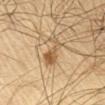Q: Is there a histopathology result?
A: catalogued during a skin exam; not biopsied
Q: Automated lesion metrics?
A: a lesion area of about 6.5 mm², an outline eccentricity of about 0.9 (0 = round, 1 = elongated), and a symmetry-axis asymmetry near 0.45; a lesion color around L≈56 a*≈16 b*≈36 in CIELAB, about 11 CIELAB-L* units darker than the surrounding skin, and a normalized border contrast of about 7.5
Q: What is the imaging modality?
A: 15 mm crop, total-body photography
Q: How large is the lesion?
A: about 4.5 mm
Q: Illumination type?
A: cross-polarized
Q: Patient demographics?
A: male, approximately 65 years of age